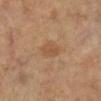The lesion was tiled from a total-body skin photograph and was not biopsied.
A roughly 15 mm field-of-view crop from a total-body skin photograph.
Located on the left lower leg.
The patient is a female aged approximately 60.